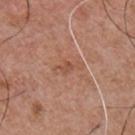Captured during whole-body skin photography for melanoma surveillance; the lesion was not biopsied. A region of skin cropped from a whole-body photographic capture, roughly 15 mm wide. This is a white-light tile. From the chest. A male subject, approximately 55 years of age. The recorded lesion diameter is about 3.5 mm.